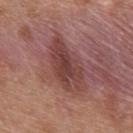Imaged during a routine full-body skin examination; the lesion was not biopsied and no histopathology is available.
An algorithmic analysis of the crop reported an outline eccentricity of about 0.85 (0 = round, 1 = elongated). It also reported a nevus-likeness score of about 0/100 and a detector confidence of about 100 out of 100 that the crop contains a lesion.
This is a white-light tile.
From the upper back.
A male subject, aged approximately 30.
About 7 mm across.
A 15 mm close-up tile from a total-body photography series done for melanoma screening.